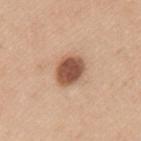biopsy_status: not biopsied; imaged during a skin examination
patient:
  sex: male
  age_approx: 35
image:
  source: total-body photography crop
  field_of_view_mm: 15
lesion_size:
  long_diameter_mm_approx: 4.0
site: arm
lighting: white-light